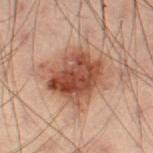No biopsy was performed on this lesion — it was imaged during a full skin examination and was not determined to be concerning. A male subject, aged approximately 55. A 15 mm close-up tile from a total-body photography series done for melanoma screening. Automated image analysis of the tile measured a shape eccentricity near 0.55 and a symmetry-axis asymmetry near 0.2. The analysis additionally found a mean CIELAB color near L≈41 a*≈20 b*≈26, about 12 CIELAB-L* units darker than the surrounding skin, and a normalized lesion–skin contrast near 9.5. And it measured a border-irregularity index near 3/10, internal color variation of about 8 on a 0–10 scale, and a peripheral color-asymmetry measure near 3. On the left thigh. Captured under cross-polarized illumination.